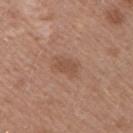Recorded during total-body skin imaging; not selected for excision or biopsy. A 15 mm close-up extracted from a 3D total-body photography capture. On the right upper arm. About 3 mm across. A female subject, in their 40s. An algorithmic analysis of the crop reported a within-lesion color-variation index near 1.5/10. And it measured a nevus-likeness score of about 15/100. Imaged with white-light lighting.